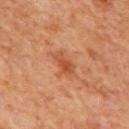Assessment: The lesion was photographed on a routine skin check and not biopsied; there is no pathology result. Acquisition and patient details: From the mid back. A male patient about 65 years old. Captured under cross-polarized illumination. A region of skin cropped from a whole-body photographic capture, roughly 15 mm wide. The total-body-photography lesion software estimated a lesion area of about 4 mm², an outline eccentricity of about 0.85 (0 = round, 1 = elongated), and a shape-asymmetry score of about 0.3 (0 = symmetric). It also reported a lesion color around L≈49 a*≈28 b*≈37 in CIELAB, a lesion–skin lightness drop of about 9, and a normalized lesion–skin contrast near 7.